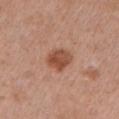This lesion was catalogued during total-body skin photography and was not selected for biopsy.
A female subject, approximately 50 years of age.
A roughly 15 mm field-of-view crop from a total-body skin photograph.
From the arm.
About 3 mm across.